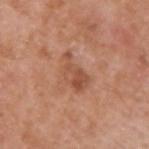{"biopsy_status": "not biopsied; imaged during a skin examination", "site": "upper back", "image": {"source": "total-body photography crop", "field_of_view_mm": 15}, "lesion_size": {"long_diameter_mm_approx": 4.5}, "patient": {"sex": "male", "age_approx": 65}, "automated_metrics": {"eccentricity": 0.85, "shape_asymmetry": 0.4, "cielab_L": 52, "cielab_a": 24, "cielab_b": 32, "vs_skin_darker_L": 8.0, "vs_skin_contrast_norm": 6.0, "border_irregularity_0_10": 4.5, "peripheral_color_asymmetry": 2.0}}